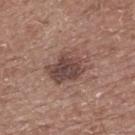Assessment: This lesion was catalogued during total-body skin photography and was not selected for biopsy. Acquisition and patient details: A close-up tile cropped from a whole-body skin photograph, about 15 mm across. The tile uses white-light illumination. The lesion's longest dimension is about 5 mm. A male subject, approximately 60 years of age. On the upper back.